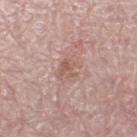The lesion was tiled from a total-body skin photograph and was not biopsied.
Approximately 3.5 mm at its widest.
Captured under white-light illumination.
A female subject in their mid- to late 60s.
A 15 mm close-up extracted from a 3D total-body photography capture.
Automated tile analysis of the lesion measured an eccentricity of roughly 0.75. And it measured a lesion color around L≈58 a*≈20 b*≈24 in CIELAB and a normalized border contrast of about 5.5. The software also gave a border-irregularity index near 3/10, a within-lesion color-variation index near 3/10, and peripheral color asymmetry of about 1.
The lesion is on the left thigh.